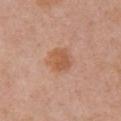The lesion was tiled from a total-body skin photograph and was not biopsied. The subject is a female aged around 60. The lesion is on the left upper arm. A roughly 15 mm field-of-view crop from a total-body skin photograph.